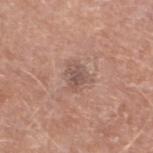No biopsy was performed on this lesion — it was imaged during a full skin examination and was not determined to be concerning. A region of skin cropped from a whole-body photographic capture, roughly 15 mm wide. The tile uses white-light illumination. Measured at roughly 2.5 mm in maximum diameter. On the left lower leg. A male subject, aged around 80.